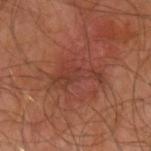| feature | finding |
|---|---|
| biopsy status | imaged on a skin check; not biopsied |
| illumination | cross-polarized |
| acquisition | total-body-photography crop, ~15 mm field of view |
| body site | the left upper arm |
| subject | male, approximately 65 years of age |
| lesion diameter | ≈5.5 mm |
| automated metrics | a footprint of about 8 mm², an eccentricity of roughly 0.95, and two-axis asymmetry of about 0.45; border irregularity of about 6.5 on a 0–10 scale, internal color variation of about 2.5 on a 0–10 scale, and a peripheral color-asymmetry measure near 1 |